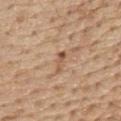The lesion was photographed on a routine skin check and not biopsied; there is no pathology result.
Cropped from a whole-body photographic skin survey; the tile spans about 15 mm.
Captured under white-light illumination.
A male patient, aged 68 to 72.
Automated tile analysis of the lesion measured roughly 10 lightness units darker than nearby skin and a lesion-to-skin contrast of about 7 (normalized; higher = more distinct).
Approximately 2.5 mm at its widest.
From the chest.